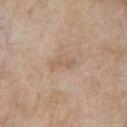Clinical impression: This lesion was catalogued during total-body skin photography and was not selected for biopsy. Context: The lesion's longest dimension is about 3 mm. A roughly 15 mm field-of-view crop from a total-body skin photograph. Located on the chest. A male patient, in their mid-60s.